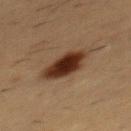workup = imaged on a skin check; not biopsied
lesion diameter = about 4.5 mm
lighting = cross-polarized illumination
image source = ~15 mm crop, total-body skin-cancer survey
patient = male, about 55 years old
site = the front of the torso